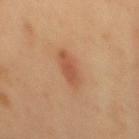Assessment:
Captured during whole-body skin photography for melanoma surveillance; the lesion was not biopsied.
Clinical summary:
The lesion is located on the back. A male patient roughly 50 years of age. The total-body-photography lesion software estimated a normalized lesion–skin contrast near 6.5. The analysis additionally found a border-irregularity index near 2.5/10, a color-variation rating of about 2/10, and peripheral color asymmetry of about 0.5. It also reported a classifier nevus-likeness of about 95/100 and a lesion-detection confidence of about 100/100. Imaged with cross-polarized lighting. This image is a 15 mm lesion crop taken from a total-body photograph. Measured at roughly 4.5 mm in maximum diameter.